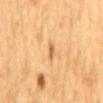This image is a 15 mm lesion crop taken from a total-body photograph.
The lesion's longest dimension is about 2.5 mm.
This is a cross-polarized tile.
The lesion is on the mid back.
A male subject aged 63 to 67.